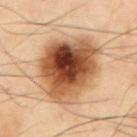Part of a total-body skin-imaging series; this lesion was reviewed on a skin check and was not flagged for biopsy. Measured at roughly 7.5 mm in maximum diameter. A 15 mm close-up tile from a total-body photography series done for melanoma screening. From the front of the torso. A male subject, aged 53–57.